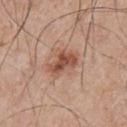biopsy status: no biopsy performed (imaged during a skin exam) | size: ≈4 mm | subject: male, roughly 55 years of age | acquisition: 15 mm crop, total-body photography | lighting: white-light illumination | site: the back.